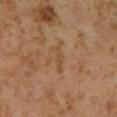Case summary:
– follow-up: catalogued during a skin exam; not biopsied
– subject: male, aged 58–62
– acquisition: ~15 mm tile from a whole-body skin photo
– site: the left lower leg